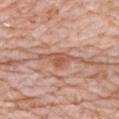  biopsy_status: not biopsied; imaged during a skin examination
  site: back
  patient:
    sex: female
    age_approx: 65
  lesion_size:
    long_diameter_mm_approx: 3.0
  automated_metrics:
    area_mm2_approx: 3.5
    eccentricity: 0.8
    shape_asymmetry: 0.45
    cielab_L: 55
    cielab_a: 25
    cielab_b: 31
    vs_skin_darker_L: 10.0
    vs_skin_contrast_norm: 7.0
  lighting: white-light
  image:
    source: total-body photography crop
    field_of_view_mm: 15